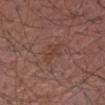Findings:
– follow-up · no biopsy performed (imaged during a skin exam)
– patient · male, aged around 55
– acquisition · ~15 mm crop, total-body skin-cancer survey
– anatomic site · the right forearm
– size · about 4 mm
– TBP lesion metrics · a lesion area of about 5.5 mm², an eccentricity of roughly 0.85, and two-axis asymmetry of about 0.35; a mean CIELAB color near L≈40 a*≈21 b*≈24, about 6 CIELAB-L* units darker than the surrounding skin, and a normalized lesion–skin contrast near 5; border irregularity of about 4.5 on a 0–10 scale, a color-variation rating of about 1.5/10, and a peripheral color-asymmetry measure near 0.5; a classifier nevus-likeness of about 0/100 and a lesion-detection confidence of about 100/100